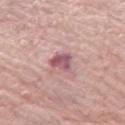biopsy_status: not biopsied; imaged during a skin examination